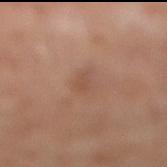Impression: No biopsy was performed on this lesion — it was imaged during a full skin examination and was not determined to be concerning. Background: A 15 mm close-up tile from a total-body photography series done for melanoma screening. This is a cross-polarized tile. Automated image analysis of the tile measured a shape eccentricity near 0.9. It also reported a lesion color around L≈49 a*≈20 b*≈29 in CIELAB. The analysis additionally found a classifier nevus-likeness of about 15/100 and a lesion-detection confidence of about 100/100. The lesion's longest dimension is about 2.5 mm. On the left leg. The subject is a male aged 48–52.